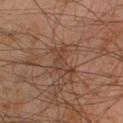{"biopsy_status": "not biopsied; imaged during a skin examination", "patient": {"sex": "male", "age_approx": 45}, "image": {"source": "total-body photography crop", "field_of_view_mm": 15}, "site": "right lower leg"}